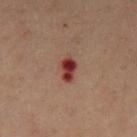No biopsy was performed on this lesion — it was imaged during a full skin examination and was not determined to be concerning. A lesion tile, about 15 mm wide, cut from a 3D total-body photograph. The lesion is located on the chest. The subject is a male in their 60s.